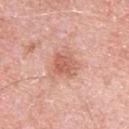site = the left upper arm | imaging modality = total-body-photography crop, ~15 mm field of view | tile lighting = white-light illumination | subject = male, aged 78–82 | diameter = ≈3 mm.